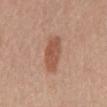Q: Was this lesion biopsied?
A: total-body-photography surveillance lesion; no biopsy
Q: Patient demographics?
A: male, roughly 65 years of age
Q: How large is the lesion?
A: ~5 mm (longest diameter)
Q: Automated lesion metrics?
A: a lesion area of about 10 mm² and a symmetry-axis asymmetry near 0.2; a mean CIELAB color near L≈53 a*≈23 b*≈30, a lesion–skin lightness drop of about 10, and a normalized lesion–skin contrast near 7.5
Q: Where on the body is the lesion?
A: the mid back
Q: Illumination type?
A: white-light illumination
Q: How was this image acquired?
A: ~15 mm tile from a whole-body skin photo